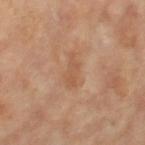Clinical impression:
Part of a total-body skin-imaging series; this lesion was reviewed on a skin check and was not flagged for biopsy.